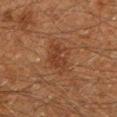Clinical impression:
Recorded during total-body skin imaging; not selected for excision or biopsy.
Acquisition and patient details:
Cropped from a total-body skin-imaging series; the visible field is about 15 mm. The lesion's longest dimension is about 4 mm. A male patient, aged around 60. Captured under cross-polarized illumination. The lesion is on the left lower leg.